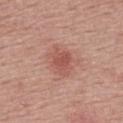<case>
<biopsy_status>not biopsied; imaged during a skin examination</biopsy_status>
</case>